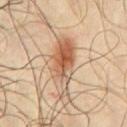Assessment: This lesion was catalogued during total-body skin photography and was not selected for biopsy. Background: The tile uses cross-polarized illumination. The lesion is on the chest. About 7 mm across. This image is a 15 mm lesion crop taken from a total-body photograph. A male patient, about 65 years old.